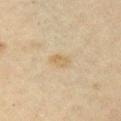The lesion was tiled from a total-body skin photograph and was not biopsied. A female patient approximately 45 years of age. The lesion is located on the left upper arm. The recorded lesion diameter is about 2.5 mm. Imaged with cross-polarized lighting. Cropped from a whole-body photographic skin survey; the tile spans about 15 mm.